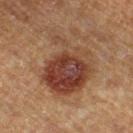Q: Was a biopsy performed?
A: catalogued during a skin exam; not biopsied
Q: Automated lesion metrics?
A: an area of roughly 32 mm², an eccentricity of roughly 0.7, and a shape-asymmetry score of about 0.4 (0 = symmetric); a lesion–skin lightness drop of about 11 and a normalized lesion–skin contrast near 9.5; a border-irregularity rating of about 5.5/10, a within-lesion color-variation index near 7.5/10, and a peripheral color-asymmetry measure near 2; a detector confidence of about 100 out of 100 that the crop contains a lesion
Q: Who is the patient?
A: male, aged approximately 65
Q: Illumination type?
A: cross-polarized illumination
Q: What is the imaging modality?
A: total-body-photography crop, ~15 mm field of view
Q: Lesion size?
A: ≈8 mm
Q: Where on the body is the lesion?
A: the right lower leg